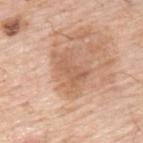workup: imaged on a skin check; not biopsied
tile lighting: white-light
lesion size: about 4 mm
image source: ~15 mm crop, total-body skin-cancer survey
location: the upper back
subject: male, aged around 70
image-analysis metrics: a lesion area of about 9 mm², an eccentricity of roughly 0.75, and a shape-asymmetry score of about 0.35 (0 = symmetric); a mean CIELAB color near L≈60 a*≈21 b*≈33; a border-irregularity index near 5/10; an automated nevus-likeness rating near 0 out of 100 and lesion-presence confidence of about 100/100